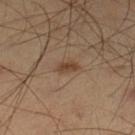Assessment: No biopsy was performed on this lesion — it was imaged during a full skin examination and was not determined to be concerning.